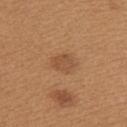Impression:
This lesion was catalogued during total-body skin photography and was not selected for biopsy.
Background:
A region of skin cropped from a whole-body photographic capture, roughly 15 mm wide. The patient is a female aged 38–42. On the left upper arm.